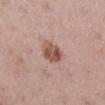Captured during whole-body skin photography for melanoma surveillance; the lesion was not biopsied.
The lesion is on the right lower leg.
A lesion tile, about 15 mm wide, cut from a 3D total-body photograph.
The subject is a female aged 53 to 57.